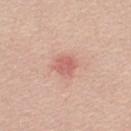| field | value |
|---|---|
| biopsy status | imaged on a skin check; not biopsied |
| lesion diameter | ≈2.5 mm |
| tile lighting | white-light |
| imaging modality | ~15 mm crop, total-body skin-cancer survey |
| location | the back |
| patient | male, aged approximately 40 |
| image-analysis metrics | an average lesion color of about L≈61 a*≈27 b*≈27 (CIELAB) and a normalized lesion–skin contrast near 6; a border-irregularity rating of about 2/10, a color-variation rating of about 2.5/10, and a peripheral color-asymmetry measure near 1 |